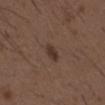Clinical impression: Recorded during total-body skin imaging; not selected for excision or biopsy. Image and clinical context: Approximately 2.5 mm at its widest. Imaged with white-light lighting. The lesion is located on the chest. A lesion tile, about 15 mm wide, cut from a 3D total-body photograph. A male patient aged around 50.